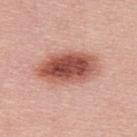{"biopsy_status": "not biopsied; imaged during a skin examination", "site": "upper back", "lesion_size": {"long_diameter_mm_approx": 7.5}, "patient": {"sex": "male", "age_approx": 30}, "lighting": "white-light", "automated_metrics": {"cielab_L": 54, "cielab_a": 28, "cielab_b": 28, "vs_skin_darker_L": 17.0, "vs_skin_contrast_norm": 11.0, "border_irregularity_0_10": 2.0, "color_variation_0_10": 8.0}, "image": {"source": "total-body photography crop", "field_of_view_mm": 15}}